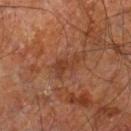Imaged during a routine full-body skin examination; the lesion was not biopsied and no histopathology is available. Located on the right leg. Captured under cross-polarized illumination. A roughly 15 mm field-of-view crop from a total-body skin photograph. An algorithmic analysis of the crop reported a mean CIELAB color near L≈38 a*≈23 b*≈31 and a lesion–skin lightness drop of about 7. It also reported a classifier nevus-likeness of about 5/100 and a detector confidence of about 100 out of 100 that the crop contains a lesion. Approximately 3.5 mm at its widest. The subject is a male roughly 60 years of age.